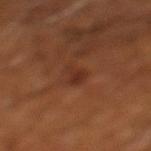Assessment: Recorded during total-body skin imaging; not selected for excision or biopsy. Acquisition and patient details: The patient is approximately 65 years of age. The recorded lesion diameter is about 3 mm. Automated tile analysis of the lesion measured an area of roughly 3.5 mm², an eccentricity of roughly 0.85, and a symmetry-axis asymmetry near 0.4. The analysis additionally found an average lesion color of about L≈31 a*≈23 b*≈29 (CIELAB), roughly 6 lightness units darker than nearby skin, and a normalized lesion–skin contrast near 6. The software also gave lesion-presence confidence of about 100/100. A lesion tile, about 15 mm wide, cut from a 3D total-body photograph. From the left lower leg.